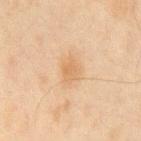The lesion was photographed on a routine skin check and not biopsied; there is no pathology result.
The lesion is on the chest.
Approximately 3 mm at its widest.
A 15 mm close-up extracted from a 3D total-body photography capture.
The patient is a male about 45 years old.